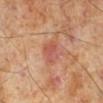  image:
    source: total-body photography crop
    field_of_view_mm: 15
  lighting: cross-polarized
  site: right lower leg
  lesion_size:
    long_diameter_mm_approx: 3.0
  patient:
    sex: male
    age_approx: 70